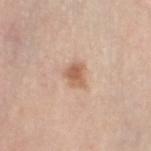{
  "lesion_size": {
    "long_diameter_mm_approx": 2.5
  },
  "patient": {
    "sex": "female",
    "age_approx": 65
  },
  "image": {
    "source": "total-body photography crop",
    "field_of_view_mm": 15
  },
  "lighting": "white-light",
  "site": "left thigh"
}